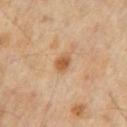biopsy status=imaged on a skin check; not biopsied
lesion size=~2.5 mm (longest diameter)
site=the mid back
patient=male, aged 63 to 67
image-analysis metrics=a lesion color around L≈54 a*≈21 b*≈36 in CIELAB and a lesion–skin lightness drop of about 11; a border-irregularity rating of about 1.5/10 and radial color variation of about 0.5
illumination=cross-polarized illumination
imaging modality=15 mm crop, total-body photography